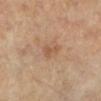A female patient in their 70s. The lesion is on the leg. This image is a 15 mm lesion crop taken from a total-body photograph. The tile uses cross-polarized illumination. Approximately 2.5 mm at its widest.